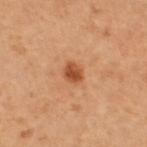Impression: The lesion was tiled from a total-body skin photograph and was not biopsied. Background: The lesion is on the left thigh. A female patient, aged 53 to 57. About 2.5 mm across. A 15 mm crop from a total-body photograph taken for skin-cancer surveillance. This is a cross-polarized tile.